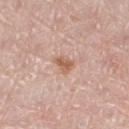Clinical impression:
No biopsy was performed on this lesion — it was imaged during a full skin examination and was not determined to be concerning.
Image and clinical context:
Captured under white-light illumination. The lesion is located on the right thigh. The patient is a female aged 63 to 67. Cropped from a whole-body photographic skin survey; the tile spans about 15 mm. The lesion's longest dimension is about 2.5 mm.